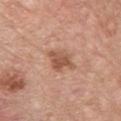| feature | finding |
|---|---|
| notes | imaged on a skin check; not biopsied |
| location | the front of the torso |
| subject | male, aged 58 to 62 |
| image | ~15 mm crop, total-body skin-cancer survey |